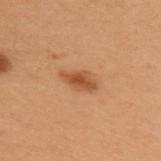Assessment: The lesion was photographed on a routine skin check and not biopsied; there is no pathology result. Acquisition and patient details: The lesion-visualizer software estimated a footprint of about 5 mm² and a symmetry-axis asymmetry near 0.25. The analysis additionally found a border-irregularity rating of about 2.5/10 and peripheral color asymmetry of about 0.5. Located on the upper back. A 15 mm close-up extracted from a 3D total-body photography capture. The recorded lesion diameter is about 3.5 mm. The tile uses cross-polarized illumination. A female subject aged 48–52.